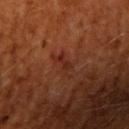The lesion was photographed on a routine skin check and not biopsied; there is no pathology result. The patient is a male in their 60s. Approximately 3 mm at its widest. This is a cross-polarized tile. A lesion tile, about 15 mm wide, cut from a 3D total-body photograph. Located on the right upper arm. Automated tile analysis of the lesion measured an area of roughly 3 mm². The analysis additionally found about 5 CIELAB-L* units darker than the surrounding skin. The software also gave a border-irregularity index near 5/10, a within-lesion color-variation index near 1.5/10, and peripheral color asymmetry of about 0.5.